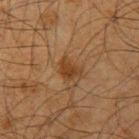workup: catalogued during a skin exam; not biopsied | TBP lesion metrics: an area of roughly 4.5 mm², an outline eccentricity of about 0.7 (0 = round, 1 = elongated), and a shape-asymmetry score of about 0.45 (0 = symmetric); an average lesion color of about L≈33 a*≈17 b*≈30 (CIELAB), roughly 7 lightness units darker than nearby skin, and a normalized border contrast of about 8; a border-irregularity rating of about 4/10, a within-lesion color-variation index near 2/10, and radial color variation of about 0.5; a lesion-detection confidence of about 100/100 | patient: male, in their mid- to late 60s | imaging modality: ~15 mm crop, total-body skin-cancer survey | lighting: cross-polarized illumination | lesion diameter: about 3 mm | body site: the arm.